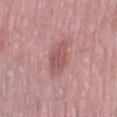follow-up=imaged on a skin check; not biopsied | patient=female, aged around 70 | lighting=white-light | lesion size=≈5 mm | image=total-body-photography crop, ~15 mm field of view | location=the right thigh.